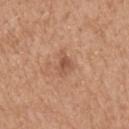Notes:
* image source: ~15 mm tile from a whole-body skin photo
* subject: male, aged 58–62
* TBP lesion metrics: an average lesion color of about L≈53 a*≈23 b*≈32 (CIELAB), roughly 9 lightness units darker than nearby skin, and a normalized border contrast of about 6.5; a color-variation rating of about 2.5/10 and a peripheral color-asymmetry measure near 0.5
* anatomic site: the head or neck
* lighting: white-light
* lesion size: about 3 mm